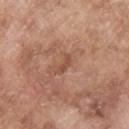workup: catalogued during a skin exam; not biopsied
imaging modality: 15 mm crop, total-body photography
tile lighting: white-light
patient: male, aged approximately 65
size: ~2.5 mm (longest diameter)
body site: the upper back